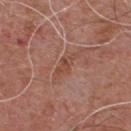No biopsy was performed on this lesion — it was imaged during a full skin examination and was not determined to be concerning. A male patient aged 53 to 57. Imaged with white-light lighting. On the chest. A close-up tile cropped from a whole-body skin photograph, about 15 mm across.